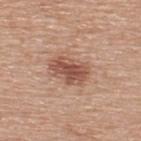* location · the upper back
* image source · ~15 mm crop, total-body skin-cancer survey
* subject · male, in their 60s